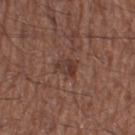Clinical impression:
No biopsy was performed on this lesion — it was imaged during a full skin examination and was not determined to be concerning.
Image and clinical context:
A male patient aged 63 to 67. A roughly 15 mm field-of-view crop from a total-body skin photograph. Automated tile analysis of the lesion measured a nevus-likeness score of about 5/100 and a detector confidence of about 100 out of 100 that the crop contains a lesion. Captured under white-light illumination. Longest diameter approximately 3 mm. Located on the right thigh.